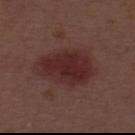<record>
<biopsy_status>not biopsied; imaged during a skin examination</biopsy_status>
<site>upper back</site>
<lighting>white-light</lighting>
<patient>
  <sex>male</sex>
  <age_approx>55</age_approx>
</patient>
<lesion_size>
  <long_diameter_mm_approx>6.5</long_diameter_mm_approx>
</lesion_size>
<image>
  <source>total-body photography crop</source>
  <field_of_view_mm>15</field_of_view_mm>
</image>
</record>